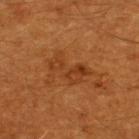No biopsy was performed on this lesion — it was imaged during a full skin examination and was not determined to be concerning. A 15 mm close-up tile from a total-body photography series done for melanoma screening. Captured under cross-polarized illumination. The lesion is located on the upper back. Approximately 5 mm at its widest. A male patient in their 60s. Automated tile analysis of the lesion measured a border-irregularity rating of about 5.5/10, internal color variation of about 4 on a 0–10 scale, and peripheral color asymmetry of about 1.5.